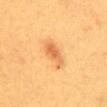Captured under cross-polarized illumination. A roughly 15 mm field-of-view crop from a total-body skin photograph. The lesion-visualizer software estimated about 9 CIELAB-L* units darker than the surrounding skin and a lesion-to-skin contrast of about 6.5 (normalized; higher = more distinct). The software also gave a classifier nevus-likeness of about 95/100 and a lesion-detection confidence of about 100/100. The lesion is on the mid back. About 4.5 mm across. A female subject, approximately 40 years of age.